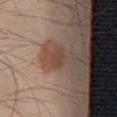Q: Was this lesion biopsied?
A: total-body-photography surveillance lesion; no biopsy
Q: Automated lesion metrics?
A: about 6 CIELAB-L* units darker than the surrounding skin and a normalized lesion–skin contrast near 5.5; lesion-presence confidence of about 100/100
Q: What is the lesion's diameter?
A: ~2.5 mm (longest diameter)
Q: Lesion location?
A: the back
Q: Who is the patient?
A: male, aged 23 to 27
Q: How was the tile lit?
A: white-light
Q: What kind of image is this?
A: 15 mm crop, total-body photography